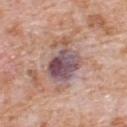biopsy status: catalogued during a skin exam; not biopsied
body site: the upper back
subject: male, aged 78–82
lighting: white-light illumination
acquisition: ~15 mm crop, total-body skin-cancer survey
automated metrics: roughly 13 lightness units darker than nearby skin and a normalized border contrast of about 10.5; a border-irregularity index near 5.5/10, a within-lesion color-variation index near 9.5/10, and a peripheral color-asymmetry measure near 3.5
size: ~6.5 mm (longest diameter)